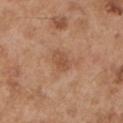No biopsy was performed on this lesion — it was imaged during a full skin examination and was not determined to be concerning. Longest diameter approximately 3 mm. A male subject aged around 55. The lesion is on the left upper arm. Cropped from a whole-body photographic skin survey; the tile spans about 15 mm.